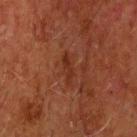Part of a total-body skin-imaging series; this lesion was reviewed on a skin check and was not flagged for biopsy. Cropped from a total-body skin-imaging series; the visible field is about 15 mm. The subject is a male aged approximately 70. The lesion is located on the head or neck. Approximately 3.5 mm at its widest. This is a cross-polarized tile.